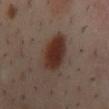Clinical impression:
Part of a total-body skin-imaging series; this lesion was reviewed on a skin check and was not flagged for biopsy.
Context:
A close-up tile cropped from a whole-body skin photograph, about 15 mm across. The subject is a male approximately 40 years of age. An algorithmic analysis of the crop reported an area of roughly 15 mm² and a symmetry-axis asymmetry near 0.15. And it measured border irregularity of about 2.5 on a 0–10 scale and a color-variation rating of about 4/10. The analysis additionally found a classifier nevus-likeness of about 100/100 and a detector confidence of about 100 out of 100 that the crop contains a lesion. The lesion is on the mid back.